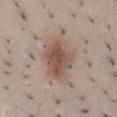Findings:
– workup — imaged on a skin check; not biopsied
– subject — female, aged 23 to 27
– size — about 5.5 mm
– image source — 15 mm crop, total-body photography
– lighting — white-light illumination
– body site — the abdomen